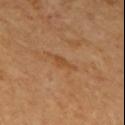Clinical summary: On the mid back. About 3 mm across. Automated tile analysis of the lesion measured a footprint of about 3 mm² and two-axis asymmetry of about 0.45. And it measured a mean CIELAB color near L≈48 a*≈22 b*≈38 and a lesion-to-skin contrast of about 5.5 (normalized; higher = more distinct). The software also gave border irregularity of about 4.5 on a 0–10 scale and peripheral color asymmetry of about 0.5. The subject is a male aged 63 to 67. A lesion tile, about 15 mm wide, cut from a 3D total-body photograph.